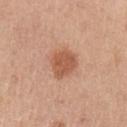Q: Is there a histopathology result?
A: imaged on a skin check; not biopsied
Q: Patient demographics?
A: female, aged around 45
Q: How was this image acquired?
A: total-body-photography crop, ~15 mm field of view
Q: Illumination type?
A: white-light
Q: What is the anatomic site?
A: the left upper arm
Q: How large is the lesion?
A: about 3.5 mm